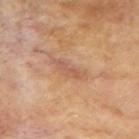Q: Was this lesion biopsied?
A: catalogued during a skin exam; not biopsied
Q: How was the tile lit?
A: cross-polarized
Q: Patient demographics?
A: female, aged 73 to 77
Q: How was this image acquired?
A: ~15 mm crop, total-body skin-cancer survey
Q: What is the anatomic site?
A: the left upper arm